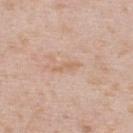<lesion>
<biopsy_status>not biopsied; imaged during a skin examination</biopsy_status>
<lighting>white-light</lighting>
<automated_metrics>
  <cielab_L>64</cielab_L>
  <cielab_a>18</cielab_a>
  <cielab_b>32</cielab_b>
  <vs_skin_darker_L>6.0</vs_skin_darker_L>
  <border_irregularity_0_10>4.5</border_irregularity_0_10>
  <color_variation_0_10>0.0</color_variation_0_10>
  <peripheral_color_asymmetry>0.0</peripheral_color_asymmetry>
  <nevus_likeness_0_100>0</nevus_likeness_0_100>
  <lesion_detection_confidence_0_100>100</lesion_detection_confidence_0_100>
</automated_metrics>
<patient>
  <sex>male</sex>
  <age_approx>40</age_approx>
</patient>
<site>back</site>
<image>
  <source>total-body photography crop</source>
  <field_of_view_mm>15</field_of_view_mm>
</image>
</lesion>